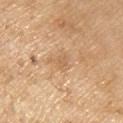The lesion was tiled from a total-body skin photograph and was not biopsied. Imaged with white-light lighting. A lesion tile, about 15 mm wide, cut from a 3D total-body photograph. A male subject approximately 70 years of age. The lesion's longest dimension is about 2.5 mm. The lesion is located on the chest.